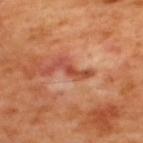This lesion was catalogued during total-body skin photography and was not selected for biopsy.
A 15 mm close-up extracted from a 3D total-body photography capture.
From the upper back.
The subject is a male aged approximately 50.